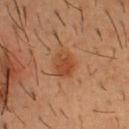notes = total-body-photography surveillance lesion; no biopsy | image = ~15 mm crop, total-body skin-cancer survey | patient = male, aged 53–57 | lighting = cross-polarized illumination | site = the chest | size = about 3 mm.